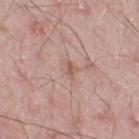<record>
  <biopsy_status>not biopsied; imaged during a skin examination</biopsy_status>
  <lighting>white-light</lighting>
  <automated_metrics>
    <border_irregularity_0_10>3.5</border_irregularity_0_10>
    <color_variation_0_10>1.5</color_variation_0_10>
    <nevus_likeness_0_100>0</nevus_likeness_0_100>
    <lesion_detection_confidence_0_100>100</lesion_detection_confidence_0_100>
  </automated_metrics>
  <lesion_size>
    <long_diameter_mm_approx>2.5</long_diameter_mm_approx>
  </lesion_size>
  <site>right thigh</site>
  <image>
    <source>total-body photography crop</source>
    <field_of_view_mm>15</field_of_view_mm>
  </image>
  <patient>
    <sex>male</sex>
    <age_approx>50</age_approx>
  </patient>
</record>